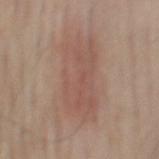Findings:
– biopsy status · catalogued during a skin exam; not biopsied
– patient · male, aged approximately 55
– tile lighting · white-light illumination
– automated metrics · a lesion color around L≈54 a*≈18 b*≈25 in CIELAB, a lesion–skin lightness drop of about 7, and a lesion-to-skin contrast of about 5 (normalized; higher = more distinct); border irregularity of about 4.5 on a 0–10 scale, a color-variation rating of about 3.5/10, and a peripheral color-asymmetry measure near 1
– diameter · ≈9 mm
– body site · the back
– imaging modality · ~15 mm crop, total-body skin-cancer survey